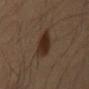Case summary:
– follow-up · no biopsy performed (imaged during a skin exam)